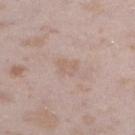Cropped from a total-body skin-imaging series; the visible field is about 15 mm. The tile uses white-light illumination. Automated tile analysis of the lesion measured a footprint of about 4 mm², an eccentricity of roughly 0.75, and two-axis asymmetry of about 0.3. And it measured about 5 CIELAB-L* units darker than the surrounding skin and a normalized border contrast of about 4.5. The lesion is on the left lower leg. The recorded lesion diameter is about 3 mm. A female patient aged approximately 25.